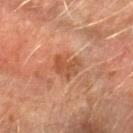<lesion>
<biopsy_status>not biopsied; imaged during a skin examination</biopsy_status>
<patient>
  <sex>male</sex>
  <age_approx>65</age_approx>
</patient>
<lighting>cross-polarized</lighting>
<site>left forearm</site>
<automated_metrics>
  <cielab_L>46</cielab_L>
  <cielab_a>23</cielab_a>
  <cielab_b>32</cielab_b>
  <vs_skin_darker_L>8.0</vs_skin_darker_L>
  <vs_skin_contrast_norm>6.5</vs_skin_contrast_norm>
  <border_irregularity_0_10>3.5</border_irregularity_0_10>
  <peripheral_color_asymmetry>1.5</peripheral_color_asymmetry>
</automated_metrics>
<image>
  <source>total-body photography crop</source>
  <field_of_view_mm>15</field_of_view_mm>
</image>
<lesion_size>
  <long_diameter_mm_approx>3.5</long_diameter_mm_approx>
</lesion_size>
</lesion>